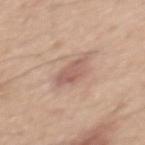No biopsy was performed on this lesion — it was imaged during a full skin examination and was not determined to be concerning. Approximately 4 mm at its widest. The lesion is located on the mid back. A male patient about 45 years old. A close-up tile cropped from a whole-body skin photograph, about 15 mm across. Imaged with white-light lighting.